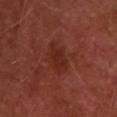Assessment:
This lesion was catalogued during total-body skin photography and was not selected for biopsy.
Clinical summary:
From the upper back. A male subject aged approximately 50. A region of skin cropped from a whole-body photographic capture, roughly 15 mm wide.